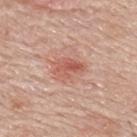Assessment: Part of a total-body skin-imaging series; this lesion was reviewed on a skin check and was not flagged for biopsy. Image and clinical context: The lesion is located on the upper back. The subject is a male aged approximately 70. A 15 mm close-up tile from a total-body photography series done for melanoma screening.